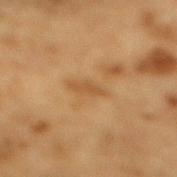Q: Is there a histopathology result?
A: no biopsy performed (imaged during a skin exam)